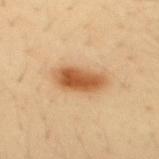Recorded during total-body skin imaging; not selected for excision or biopsy. A 15 mm crop from a total-body photograph taken for skin-cancer surveillance. A female subject, in their mid- to late 30s. This is a cross-polarized tile. From the upper back.